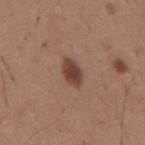{"biopsy_status": "not biopsied; imaged during a skin examination", "image": {"source": "total-body photography crop", "field_of_view_mm": 15}, "site": "back", "lighting": "white-light", "patient": {"sex": "male", "age_approx": 65}}